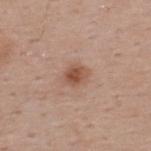biopsy status: total-body-photography surveillance lesion; no biopsy
automated metrics: a symmetry-axis asymmetry near 0.2; a classifier nevus-likeness of about 90/100 and a detector confidence of about 100 out of 100 that the crop contains a lesion
image: ~15 mm tile from a whole-body skin photo
patient: male, aged 38–42
lesion diameter: about 2.5 mm
body site: the upper back